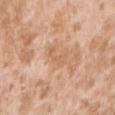Impression: Recorded during total-body skin imaging; not selected for excision or biopsy. Image and clinical context: The lesion is located on the right upper arm. A female subject approximately 25 years of age. Cropped from a whole-body photographic skin survey; the tile spans about 15 mm.